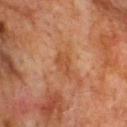Q: How large is the lesion?
A: ~3 mm (longest diameter)
Q: What kind of image is this?
A: total-body-photography crop, ~15 mm field of view
Q: What is the anatomic site?
A: the upper back
Q: How was the tile lit?
A: cross-polarized illumination
Q: What are the patient's age and sex?
A: male, aged around 75